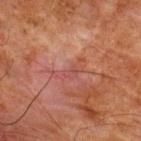Background:
This is a cross-polarized tile. The lesion is on the upper back. Cropped from a whole-body photographic skin survey; the tile spans about 15 mm. The recorded lesion diameter is about 4 mm. An algorithmic analysis of the crop reported an average lesion color of about L≈39 a*≈23 b*≈23 (CIELAB), roughly 5 lightness units darker than nearby skin, and a normalized lesion–skin contrast near 5. The patient is a male aged 63 to 67.